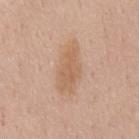Imaged during a routine full-body skin examination; the lesion was not biopsied and no histopathology is available.
A region of skin cropped from a whole-body photographic capture, roughly 15 mm wide.
The lesion is located on the chest.
The lesion's longest dimension is about 6 mm.
An algorithmic analysis of the crop reported a mean CIELAB color near L≈61 a*≈18 b*≈32. And it measured a color-variation rating of about 2/10 and peripheral color asymmetry of about 0.5.
Captured under white-light illumination.
A male patient, aged 53 to 57.